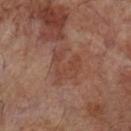Assessment:
No biopsy was performed on this lesion — it was imaged during a full skin examination and was not determined to be concerning.
Acquisition and patient details:
This is a cross-polarized tile. The subject is a male roughly 70 years of age. This image is a 15 mm lesion crop taken from a total-body photograph. The lesion is on the left lower leg. About 4.5 mm across.